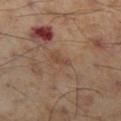This lesion was catalogued during total-body skin photography and was not selected for biopsy. A roughly 15 mm field-of-view crop from a total-body skin photograph. Measured at roughly 2.5 mm in maximum diameter. A male patient, approximately 55 years of age. The lesion is located on the left thigh. Automated image analysis of the tile measured an area of roughly 2.5 mm², an outline eccentricity of about 0.9 (0 = round, 1 = elongated), and a shape-asymmetry score of about 0.35 (0 = symmetric). The analysis additionally found a border-irregularity index near 3.5/10, a within-lesion color-variation index near 0/10, and peripheral color asymmetry of about 0. The analysis additionally found an automated nevus-likeness rating near 0 out of 100 and a lesion-detection confidence of about 100/100. Captured under cross-polarized illumination.